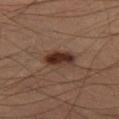biopsy status: imaged on a skin check; not biopsied | body site: the leg | subject: male, aged 18 to 22 | image: total-body-photography crop, ~15 mm field of view.